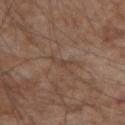The lesion was tiled from a total-body skin photograph and was not biopsied. The lesion is located on the right forearm. Approximately 3 mm at its widest. Automated image analysis of the tile measured an area of roughly 2.5 mm² and an outline eccentricity of about 0.9 (0 = round, 1 = elongated). The analysis additionally found roughly 6 lightness units darker than nearby skin and a normalized lesion–skin contrast near 5. This image is a 15 mm lesion crop taken from a total-body photograph. A male subject, in their mid-70s. Imaged with white-light lighting.